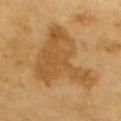Clinical impression:
The lesion was photographed on a routine skin check and not biopsied; there is no pathology result.
Background:
The subject is a male approximately 85 years of age or older. Captured under cross-polarized illumination. Automated image analysis of the tile measured a lesion area of about 35 mm², an eccentricity of roughly 0.6, and two-axis asymmetry of about 0.6. It also reported a detector confidence of about 95 out of 100 that the crop contains a lesion. A close-up tile cropped from a whole-body skin photograph, about 15 mm across. Measured at roughly 9 mm in maximum diameter. The lesion is located on the chest.